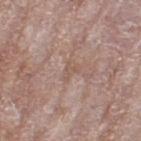The lesion was photographed on a routine skin check and not biopsied; there is no pathology result.
A 15 mm close-up extracted from a 3D total-body photography capture.
The recorded lesion diameter is about 2.5 mm.
The subject is a female approximately 75 years of age.
Automated image analysis of the tile measured a footprint of about 3 mm², a shape eccentricity near 0.8, and two-axis asymmetry of about 0.55.
On the left thigh.